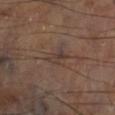{
  "biopsy_status": "not biopsied; imaged during a skin examination",
  "lesion_size": {
    "long_diameter_mm_approx": 2.5
  },
  "site": "leg",
  "automated_metrics": {
    "area_mm2_approx": 3.5,
    "eccentricity": 0.75,
    "border_irregularity_0_10": 3.5,
    "color_variation_0_10": 4.0,
    "peripheral_color_asymmetry": 1.5,
    "nevus_likeness_0_100": 0,
    "lesion_detection_confidence_0_100": 75
  },
  "image": {
    "source": "total-body photography crop",
    "field_of_view_mm": 15
  }
}